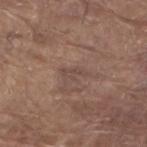Findings:
• workup · total-body-photography surveillance lesion; no biopsy
• acquisition · ~15 mm crop, total-body skin-cancer survey
• tile lighting · white-light
• location · the right upper arm
• patient · male, roughly 80 years of age
• image-analysis metrics · a lesion area of about 2.5 mm², an eccentricity of roughly 0.9, and a symmetry-axis asymmetry near 0.45; a border-irregularity rating of about 5.5/10, internal color variation of about 0 on a 0–10 scale, and peripheral color asymmetry of about 0